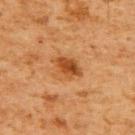Notes:
• illumination · cross-polarized illumination
• acquisition · ~15 mm tile from a whole-body skin photo
• location · the upper back
• subject · male, approximately 60 years of age